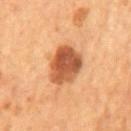<lesion>
<biopsy_status>not biopsied; imaged during a skin examination</biopsy_status>
<lighting>cross-polarized</lighting>
<patient>
  <sex>male</sex>
  <age_approx>55</age_approx>
</patient>
<image>
  <source>total-body photography crop</source>
  <field_of_view_mm>15</field_of_view_mm>
</image>
<site>mid back</site>
<automated_metrics>
  <area_mm2_approx>13.0</area_mm2_approx>
  <eccentricity>0.75</eccentricity>
  <shape_asymmetry>0.2</shape_asymmetry>
  <cielab_L>50</cielab_L>
  <cielab_a>26</cielab_a>
  <cielab_b>37</cielab_b>
  <vs_skin_darker_L>16.0</vs_skin_darker_L>
  <vs_skin_contrast_norm>10.5</vs_skin_contrast_norm>
  <lesion_detection_confidence_0_100>100</lesion_detection_confidence_0_100>
</automated_metrics>
<lesion_size>
  <long_diameter_mm_approx>5.0</long_diameter_mm_approx>
</lesion_size>
</lesion>